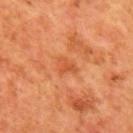{
  "biopsy_status": "not biopsied; imaged during a skin examination",
  "site": "back",
  "patient": {
    "sex": "male",
    "age_approx": 65
  },
  "image": {
    "source": "total-body photography crop",
    "field_of_view_mm": 15
  }
}